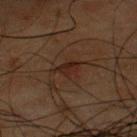biopsy status = catalogued during a skin exam; not biopsied
acquisition = total-body-photography crop, ~15 mm field of view
lighting = cross-polarized
patient = male, roughly 50 years of age
lesion size = about 2.5 mm
site = the chest